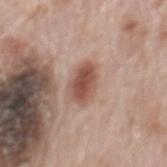notes = catalogued during a skin exam; not biopsied | subject = male, aged approximately 70 | image-analysis metrics = a mean CIELAB color near L≈52 a*≈20 b*≈27 and a normalized lesion–skin contrast near 8.5; a nevus-likeness score of about 95/100 and a lesion-detection confidence of about 100/100 | image source = total-body-photography crop, ~15 mm field of view | site = the back.